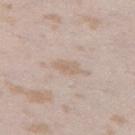follow-up: catalogued during a skin exam; not biopsied | size: about 3.5 mm | site: the leg | lighting: white-light illumination | patient: female, roughly 25 years of age | image: 15 mm crop, total-body photography | automated metrics: a mean CIELAB color near L≈64 a*≈14 b*≈26, roughly 6 lightness units darker than nearby skin, and a lesion-to-skin contrast of about 5 (normalized; higher = more distinct); internal color variation of about 1 on a 0–10 scale.